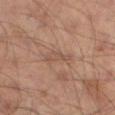Clinical impression:
Recorded during total-body skin imaging; not selected for excision or biopsy.
Image and clinical context:
The lesion-visualizer software estimated a border-irregularity rating of about 4/10, a within-lesion color-variation index near 1/10, and peripheral color asymmetry of about 0.5. And it measured an automated nevus-likeness rating near 0 out of 100 and a detector confidence of about 95 out of 100 that the crop contains a lesion. The tile uses cross-polarized illumination. Measured at roughly 3.5 mm in maximum diameter. A male subject, aged 53 to 57. Located on the left thigh. A region of skin cropped from a whole-body photographic capture, roughly 15 mm wide.